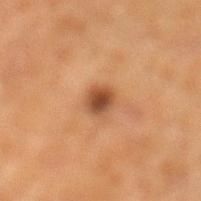This lesion was catalogued during total-body skin photography and was not selected for biopsy.
A male patient aged 63–67.
The lesion's longest dimension is about 2.5 mm.
This is a cross-polarized tile.
This image is a 15 mm lesion crop taken from a total-body photograph.
From the left lower leg.